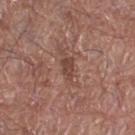Part of a total-body skin-imaging series; this lesion was reviewed on a skin check and was not flagged for biopsy.
Automated image analysis of the tile measured a mean CIELAB color near L≈43 a*≈20 b*≈24 and roughly 9 lightness units darker than nearby skin. And it measured a border-irregularity index near 3.5/10 and a color-variation rating of about 1.5/10.
The subject is a male about 75 years old.
The lesion is located on the right thigh.
A close-up tile cropped from a whole-body skin photograph, about 15 mm across.
Imaged with white-light lighting.
The lesion's longest dimension is about 2.5 mm.